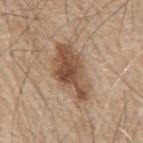workup = no biopsy performed (imaged during a skin exam) | patient = male, about 80 years old | body site = the mid back | image = ~15 mm crop, total-body skin-cancer survey.